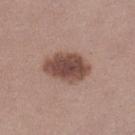Clinical impression: Captured during whole-body skin photography for melanoma surveillance; the lesion was not biopsied. Clinical summary: Cropped from a total-body skin-imaging series; the visible field is about 15 mm. About 5 mm across. The tile uses white-light illumination. A female subject, about 30 years old. The lesion is located on the leg.